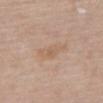Q: Was a biopsy performed?
A: no biopsy performed (imaged during a skin exam)
Q: Lesion location?
A: the back
Q: Automated lesion metrics?
A: an outline eccentricity of about 0.95 (0 = round, 1 = elongated) and a shape-asymmetry score of about 0.3 (0 = symmetric); a nevus-likeness score of about 0/100
Q: What is the imaging modality?
A: ~15 mm crop, total-body skin-cancer survey
Q: Patient demographics?
A: female, about 65 years old
Q: How large is the lesion?
A: ≈4.5 mm